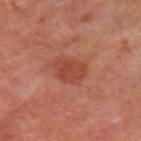A close-up tile cropped from a whole-body skin photograph, about 15 mm across. A male subject, in their mid- to late 60s. The lesion is located on the left upper arm. The recorded lesion diameter is about 3.5 mm.